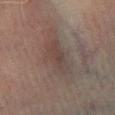Clinical impression:
Part of a total-body skin-imaging series; this lesion was reviewed on a skin check and was not flagged for biopsy.
Context:
From the left lower leg. A male patient, aged approximately 70. The recorded lesion diameter is about 4.5 mm. A 15 mm crop from a total-body photograph taken for skin-cancer surveillance. Imaged with cross-polarized lighting.